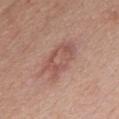workup — total-body-photography surveillance lesion; no biopsy | size — ~5.5 mm (longest diameter) | subject — male, aged approximately 50 | automated metrics — a footprint of about 12 mm² and two-axis asymmetry of about 0.2; a mean CIELAB color near L≈54 a*≈23 b*≈25 and a lesion-to-skin contrast of about 5.5 (normalized; higher = more distinct); a border-irregularity rating of about 3.5/10, internal color variation of about 3.5 on a 0–10 scale, and radial color variation of about 1 | site — the chest | acquisition — 15 mm crop, total-body photography.